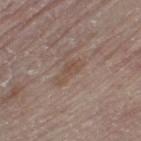notes: total-body-photography surveillance lesion; no biopsy | imaging modality: total-body-photography crop, ~15 mm field of view | patient: male, aged approximately 80 | anatomic site: the leg.